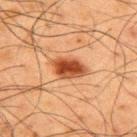Recorded during total-body skin imaging; not selected for excision or biopsy. Measured at roughly 4 mm in maximum diameter. An algorithmic analysis of the crop reported a lesion color around L≈39 a*≈24 b*≈33 in CIELAB and a lesion-to-skin contrast of about 11 (normalized; higher = more distinct). The software also gave a border-irregularity rating of about 1.5/10, internal color variation of about 4.5 on a 0–10 scale, and a peripheral color-asymmetry measure near 1.5. The analysis additionally found a classifier nevus-likeness of about 100/100 and a lesion-detection confidence of about 100/100. A region of skin cropped from a whole-body photographic capture, roughly 15 mm wide. On the upper back. A male subject, aged around 60.